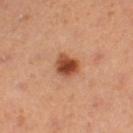Clinical impression:
Recorded during total-body skin imaging; not selected for excision or biopsy.
Acquisition and patient details:
Cropped from a whole-body photographic skin survey; the tile spans about 15 mm. The lesion is located on the left thigh. The subject is a female in their mid- to late 30s.